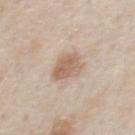Imaged during a routine full-body skin examination; the lesion was not biopsied and no histopathology is available. On the chest. A roughly 15 mm field-of-view crop from a total-body skin photograph. The total-body-photography lesion software estimated a footprint of about 8.5 mm², a shape eccentricity near 0.5, and a shape-asymmetry score of about 0.3 (0 = symmetric). The tile uses white-light illumination. Longest diameter approximately 3.5 mm. A male patient, in their 60s.